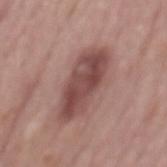No biopsy was performed on this lesion — it was imaged during a full skin examination and was not determined to be concerning.
About 7.5 mm across.
Cropped from a total-body skin-imaging series; the visible field is about 15 mm.
Located on the mid back.
The subject is a male in their mid- to late 70s.
The tile uses white-light illumination.